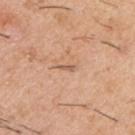workup=no biopsy performed (imaged during a skin exam)